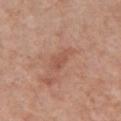The lesion was photographed on a routine skin check and not biopsied; there is no pathology result. The tile uses white-light illumination. The lesion's longest dimension is about 3.5 mm. The lesion is located on the chest. The subject is a female roughly 60 years of age. The lesion-visualizer software estimated an outline eccentricity of about 0.9 (0 = round, 1 = elongated). The analysis additionally found a mean CIELAB color near L≈53 a*≈23 b*≈29, roughly 7 lightness units darker than nearby skin, and a normalized border contrast of about 5. A 15 mm close-up tile from a total-body photography series done for melanoma screening.